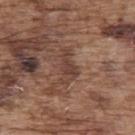Q: Was a biopsy performed?
A: total-body-photography surveillance lesion; no biopsy
Q: Patient demographics?
A: male, aged around 75
Q: What is the imaging modality?
A: ~15 mm tile from a whole-body skin photo
Q: Lesion location?
A: the upper back
Q: How was the tile lit?
A: white-light illumination
Q: What is the lesion's diameter?
A: ~4.5 mm (longest diameter)